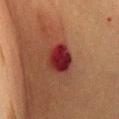notes — imaged on a skin check; not biopsied
image-analysis metrics — a within-lesion color-variation index near 5.5/10 and a peripheral color-asymmetry measure near 1.5; a nevus-likeness score of about 0/100 and a lesion-detection confidence of about 100/100
tile lighting — cross-polarized illumination
body site — the chest
diameter — about 4 mm
acquisition — ~15 mm tile from a whole-body skin photo
subject — female, roughly 55 years of age